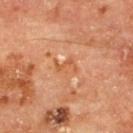biopsy_status: not biopsied; imaged during a skin examination
patient:
  sex: male
  age_approx: 65
image:
  source: total-body photography crop
  field_of_view_mm: 15
lighting: cross-polarized
site: upper back